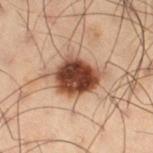Part of a total-body skin-imaging series; this lesion was reviewed on a skin check and was not flagged for biopsy. A 15 mm crop from a total-body photograph taken for skin-cancer surveillance. A male patient, about 55 years old. Captured under cross-polarized illumination. The recorded lesion diameter is about 5 mm. From the leg.